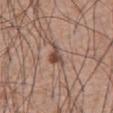Approximately 3 mm at its widest. The subject is a male aged 58–62. Cropped from a total-body skin-imaging series; the visible field is about 15 mm. The total-body-photography lesion software estimated a lesion area of about 3.5 mm², an outline eccentricity of about 0.8 (0 = round, 1 = elongated), and a symmetry-axis asymmetry near 0.5. And it measured a lesion color around L≈46 a*≈19 b*≈25 in CIELAB and a lesion–skin lightness drop of about 12. And it measured a detector confidence of about 100 out of 100 that the crop contains a lesion. Captured under white-light illumination. The lesion is on the abdomen.